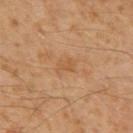Part of a total-body skin-imaging series; this lesion was reviewed on a skin check and was not flagged for biopsy.
A roughly 15 mm field-of-view crop from a total-body skin photograph.
The tile uses cross-polarized illumination.
Located on the mid back.
A male patient, approximately 60 years of age.